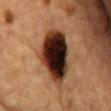Q: Is there a histopathology result?
A: no biopsy performed (imaged during a skin exam)
Q: Lesion size?
A: about 8 mm
Q: Lesion location?
A: the chest
Q: Automated lesion metrics?
A: a lesion area of about 26 mm², a shape eccentricity near 0.8, and a shape-asymmetry score of about 0.15 (0 = symmetric); a mean CIELAB color near L≈25 a*≈18 b*≈22, a lesion–skin lightness drop of about 23, and a lesion-to-skin contrast of about 20.5 (normalized; higher = more distinct)
Q: What lighting was used for the tile?
A: cross-polarized illumination
Q: Patient demographics?
A: male, aged approximately 85 or older
Q: What kind of image is this?
A: ~15 mm tile from a whole-body skin photo